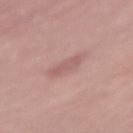Imaged during a routine full-body skin examination; the lesion was not biopsied and no histopathology is available. Cropped from a total-body skin-imaging series; the visible field is about 15 mm. On the left thigh. Automated tile analysis of the lesion measured a mean CIELAB color near L≈57 a*≈23 b*≈22, roughly 7 lightness units darker than nearby skin, and a normalized lesion–skin contrast near 5. A male subject aged approximately 70. The tile uses white-light illumination.